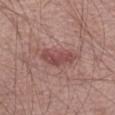image source: ~15 mm crop, total-body skin-cancer survey; diameter: about 4.5 mm; subject: male, approximately 65 years of age; tile lighting: white-light illumination; location: the leg.